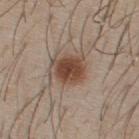Part of a total-body skin-imaging series; this lesion was reviewed on a skin check and was not flagged for biopsy.
Automated tile analysis of the lesion measured an average lesion color of about L≈46 a*≈17 b*≈27 (CIELAB) and a lesion-to-skin contrast of about 10 (normalized; higher = more distinct).
A male subject aged around 30.
The lesion is on the upper back.
The tile uses white-light illumination.
A close-up tile cropped from a whole-body skin photograph, about 15 mm across.
About 4.5 mm across.